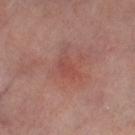<case>
<lighting>cross-polarized</lighting>
<patient>
  <sex>female</sex>
  <age_approx>50</age_approx>
</patient>
<image>
  <source>total-body photography crop</source>
  <field_of_view_mm>15</field_of_view_mm>
</image>
<site>right thigh</site>
<lesion_size>
  <long_diameter_mm_approx>4.0</long_diameter_mm_approx>
</lesion_size>
</case>